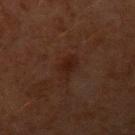Assessment: Imaged during a routine full-body skin examination; the lesion was not biopsied and no histopathology is available. Context: About 3 mm across. Cropped from a whole-body photographic skin survey; the tile spans about 15 mm. The patient is a male aged 58–62. Located on the left upper arm. Imaged with cross-polarized lighting.